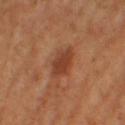Impression:
This lesion was catalogued during total-body skin photography and was not selected for biopsy.
Image and clinical context:
On the right thigh. The tile uses cross-polarized illumination. The patient is a female about 55 years old. Automated tile analysis of the lesion measured a lesion area of about 7.5 mm² and a shape-asymmetry score of about 0.25 (0 = symmetric). The software also gave internal color variation of about 3 on a 0–10 scale and peripheral color asymmetry of about 1. The software also gave a nevus-likeness score of about 35/100 and a detector confidence of about 100 out of 100 that the crop contains a lesion. The lesion's longest dimension is about 4 mm. A close-up tile cropped from a whole-body skin photograph, about 15 mm across.